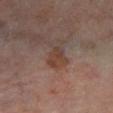notes — imaged on a skin check; not biopsied
automated lesion analysis — an area of roughly 4 mm² and a shape-asymmetry score of about 0.3 (0 = symmetric); a nevus-likeness score of about 0/100
anatomic site — the left lower leg
diameter — about 3 mm
subject — male, aged approximately 70
tile lighting — cross-polarized illumination
image — ~15 mm tile from a whole-body skin photo